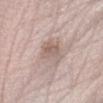illumination: white-light illumination
patient: male, in their mid- to late 60s
location: the right forearm
image-analysis metrics: a border-irregularity index near 2.5/10, a color-variation rating of about 3.5/10, and radial color variation of about 1; a nevus-likeness score of about 5/100 and a lesion-detection confidence of about 55/100
acquisition: ~15 mm crop, total-body skin-cancer survey
lesion size: about 4.5 mm